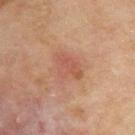Clinical impression: Captured during whole-body skin photography for melanoma surveillance; the lesion was not biopsied. Background: Measured at roughly 4 mm in maximum diameter. A male patient, aged 43–47. A lesion tile, about 15 mm wide, cut from a 3D total-body photograph. Imaged with cross-polarized lighting. From the upper back. Automated image analysis of the tile measured an outline eccentricity of about 0.5 (0 = round, 1 = elongated) and two-axis asymmetry of about 0.3. The analysis additionally found a mean CIELAB color near L≈47 a*≈21 b*≈26, roughly 6 lightness units darker than nearby skin, and a lesion-to-skin contrast of about 5 (normalized; higher = more distinct).